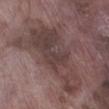follow-up: catalogued during a skin exam; not biopsied
diameter: ~10.5 mm (longest diameter)
TBP lesion metrics: an area of roughly 43 mm², a shape eccentricity near 0.8, and a shape-asymmetry score of about 0.5 (0 = symmetric); a mean CIELAB color near L≈41 a*≈16 b*≈17, about 9 CIELAB-L* units darker than the surrounding skin, and a lesion-to-skin contrast of about 7.5 (normalized; higher = more distinct); a border-irregularity rating of about 8/10, a within-lesion color-variation index near 5.5/10, and a peripheral color-asymmetry measure near 2
patient: male, in their mid-70s
body site: the right lower leg
imaging modality: total-body-photography crop, ~15 mm field of view